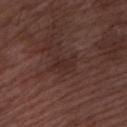workup: total-body-photography surveillance lesion; no biopsy
patient: male, in their mid-70s
illumination: white-light
location: the left forearm
imaging modality: ~15 mm crop, total-body skin-cancer survey
lesion size: about 3 mm
TBP lesion metrics: a footprint of about 4 mm² and an outline eccentricity of about 0.85 (0 = round, 1 = elongated)